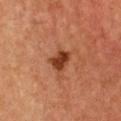Impression:
Part of a total-body skin-imaging series; this lesion was reviewed on a skin check and was not flagged for biopsy.
Acquisition and patient details:
Automated image analysis of the tile measured a classifier nevus-likeness of about 90/100 and a detector confidence of about 100 out of 100 that the crop contains a lesion. The subject is a female roughly 60 years of age. The recorded lesion diameter is about 2.5 mm. Imaged with cross-polarized lighting. The lesion is located on the chest. A lesion tile, about 15 mm wide, cut from a 3D total-body photograph.